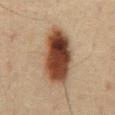{
  "lesion_size": {
    "long_diameter_mm_approx": 7.5
  },
  "patient": {
    "sex": "male",
    "age_approx": 60
  },
  "image": {
    "source": "total-body photography crop",
    "field_of_view_mm": 15
  },
  "site": "abdomen",
  "diagnosis": {
    "histopathology": "junctional melanocytic nevus",
    "malignancy": "benign",
    "taxonomic_path": [
      "Benign",
      "Benign melanocytic proliferations",
      "Nevus",
      "Nevus, NOS, Junctional"
    ]
  }
}